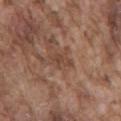follow-up=total-body-photography surveillance lesion; no biopsy
subject=male, aged approximately 75
site=the mid back
lighting=white-light illumination
size=≈3 mm
image source=total-body-photography crop, ~15 mm field of view